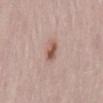Q: Was this lesion biopsied?
A: imaged on a skin check; not biopsied
Q: What kind of image is this?
A: ~15 mm tile from a whole-body skin photo
Q: Lesion location?
A: the mid back
Q: What is the lesion's diameter?
A: about 2.5 mm
Q: Who is the patient?
A: female, aged 28 to 32